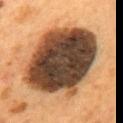Impression:
No biopsy was performed on this lesion — it was imaged during a full skin examination and was not determined to be concerning.
Image and clinical context:
Located on the mid back. A male subject, about 55 years old. The tile uses cross-polarized illumination. Approximately 9.5 mm at its widest. A lesion tile, about 15 mm wide, cut from a 3D total-body photograph.